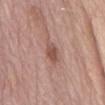A close-up tile cropped from a whole-body skin photograph, about 15 mm across.
A male patient, roughly 70 years of age.
Located on the abdomen.